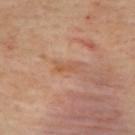Imaged during a routine full-body skin examination; the lesion was not biopsied and no histopathology is available. A female patient, aged approximately 40. The lesion is located on the upper back. A lesion tile, about 15 mm wide, cut from a 3D total-body photograph. An algorithmic analysis of the crop reported a footprint of about 3 mm², an outline eccentricity of about 0.95 (0 = round, 1 = elongated), and two-axis asymmetry of about 0.25. And it measured a lesion color around L≈56 a*≈22 b*≈34 in CIELAB, a lesion–skin lightness drop of about 7, and a normalized border contrast of about 5.5. The software also gave a border-irregularity index near 3.5/10, internal color variation of about 0 on a 0–10 scale, and radial color variation of about 0. The analysis additionally found an automated nevus-likeness rating near 0 out of 100 and a lesion-detection confidence of about 100/100. Measured at roughly 2.5 mm in maximum diameter.